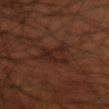Findings:
* notes: imaged on a skin check; not biopsied
* lighting: cross-polarized illumination
* patient: male, in their 50s
* image-analysis metrics: an area of roughly 7 mm², an outline eccentricity of about 0.8 (0 = round, 1 = elongated), and a symmetry-axis asymmetry near 0.35; a mean CIELAB color near L≈17 a*≈15 b*≈19; lesion-presence confidence of about 90/100
* anatomic site: the right forearm
* image source: total-body-photography crop, ~15 mm field of view